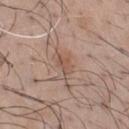Q: Was this lesion biopsied?
A: total-body-photography surveillance lesion; no biopsy
Q: Lesion location?
A: the chest
Q: How was this image acquired?
A: 15 mm crop, total-body photography
Q: What lighting was used for the tile?
A: white-light illumination
Q: What are the patient's age and sex?
A: male, about 45 years old
Q: What did automated image analysis measure?
A: a symmetry-axis asymmetry near 0.25; a nevus-likeness score of about 5/100 and a detector confidence of about 100 out of 100 that the crop contains a lesion